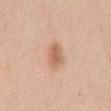Q: Was a biopsy performed?
A: imaged on a skin check; not biopsied
Q: How large is the lesion?
A: ≈3 mm
Q: Where on the body is the lesion?
A: the abdomen
Q: Who is the patient?
A: female, aged 33–37
Q: What kind of image is this?
A: total-body-photography crop, ~15 mm field of view
Q: Automated lesion metrics?
A: an average lesion color of about L≈62 a*≈21 b*≈33 (CIELAB), roughly 11 lightness units darker than nearby skin, and a normalized border contrast of about 7; a border-irregularity index near 2/10, a color-variation rating of about 3/10, and a peripheral color-asymmetry measure near 1; a nevus-likeness score of about 95/100 and lesion-presence confidence of about 100/100